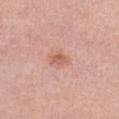| feature | finding |
|---|---|
| biopsy status | catalogued during a skin exam; not biopsied |
| image source | ~15 mm crop, total-body skin-cancer survey |
| lesion size | ≈2.5 mm |
| body site | the abdomen |
| patient | female, aged 38–42 |
| tile lighting | white-light illumination |